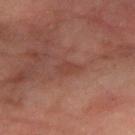Impression: The lesion was tiled from a total-body skin photograph and was not biopsied. Context: Automated image analysis of the tile measured a lesion–skin lightness drop of about 5 and a lesion-to-skin contrast of about 5 (normalized; higher = more distinct). And it measured a border-irregularity rating of about 2.5/10, a color-variation rating of about 0.5/10, and radial color variation of about 0. A male subject, approximately 60 years of age. The lesion is located on the left forearm. Cropped from a whole-body photographic skin survey; the tile spans about 15 mm.